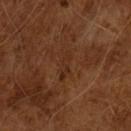This lesion was catalogued during total-body skin photography and was not selected for biopsy. The recorded lesion diameter is about 3.5 mm. A male subject, aged 63 to 67. Captured under cross-polarized illumination. The total-body-photography lesion software estimated a lesion color around L≈27 a*≈20 b*≈28 in CIELAB and a lesion-to-skin contrast of about 5.5 (normalized; higher = more distinct). It also reported lesion-presence confidence of about 85/100. Cropped from a whole-body photographic skin survey; the tile spans about 15 mm.